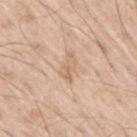Clinical impression: This lesion was catalogued during total-body skin photography and was not selected for biopsy. Clinical summary: The subject is a male approximately 70 years of age. Longest diameter approximately 3 mm. Imaged with white-light lighting. The lesion is on the right upper arm. An algorithmic analysis of the crop reported border irregularity of about 4 on a 0–10 scale, a color-variation rating of about 3/10, and peripheral color asymmetry of about 1. It also reported a detector confidence of about 100 out of 100 that the crop contains a lesion. A close-up tile cropped from a whole-body skin photograph, about 15 mm across.